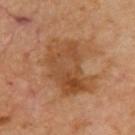Imaged during a routine full-body skin examination; the lesion was not biopsied and no histopathology is available. Measured at roughly 7 mm in maximum diameter. The lesion-visualizer software estimated a lesion color around L≈47 a*≈22 b*≈36 in CIELAB, a lesion–skin lightness drop of about 9, and a lesion-to-skin contrast of about 7 (normalized; higher = more distinct). And it measured border irregularity of about 4 on a 0–10 scale. The lesion is located on the upper back. A patient in their mid-60s. This is a cross-polarized tile. A roughly 15 mm field-of-view crop from a total-body skin photograph.